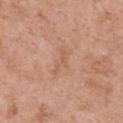Impression:
The lesion was tiled from a total-body skin photograph and was not biopsied.
Acquisition and patient details:
Longest diameter approximately 3.5 mm. On the chest. This image is a 15 mm lesion crop taken from a total-body photograph. A female subject, approximately 50 years of age. Imaged with white-light lighting.